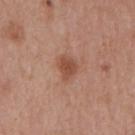Case summary:
– biopsy status — no biopsy performed (imaged during a skin exam)
– subject — male, about 70 years old
– acquisition — 15 mm crop, total-body photography
– tile lighting — white-light
– automated lesion analysis — a lesion color around L≈49 a*≈23 b*≈30 in CIELAB, about 10 CIELAB-L* units darker than the surrounding skin, and a lesion-to-skin contrast of about 7.5 (normalized; higher = more distinct); a border-irregularity index near 2.5/10 and internal color variation of about 1.5 on a 0–10 scale
– site — the mid back